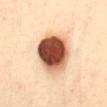Recorded during total-body skin imaging; not selected for excision or biopsy.